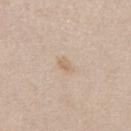| field | value |
|---|---|
| biopsy status | total-body-photography surveillance lesion; no biopsy |
| automated metrics | an eccentricity of roughly 0.85 and two-axis asymmetry of about 0.35; an average lesion color of about L≈66 a*≈14 b*≈31 (CIELAB) and a lesion–skin lightness drop of about 7; a border-irregularity rating of about 3/10, a color-variation rating of about 1.5/10, and a peripheral color-asymmetry measure near 0.5 |
| lesion diameter | about 2.5 mm |
| acquisition | ~15 mm crop, total-body skin-cancer survey |
| tile lighting | white-light illumination |
| subject | female, aged around 45 |
| anatomic site | the back |